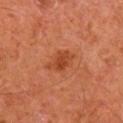No biopsy was performed on this lesion — it was imaged during a full skin examination and was not determined to be concerning. A 15 mm close-up extracted from a 3D total-body photography capture. A male patient, about 65 years old. Measured at roughly 3.5 mm in maximum diameter. The lesion-visualizer software estimated border irregularity of about 3.5 on a 0–10 scale, a color-variation rating of about 2.5/10, and peripheral color asymmetry of about 0.5. The software also gave an automated nevus-likeness rating near 55 out of 100. Captured under cross-polarized illumination. On the right upper arm.